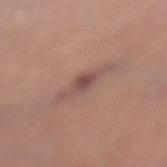Assessment: No biopsy was performed on this lesion — it was imaged during a full skin examination and was not determined to be concerning. Background: The lesion-visualizer software estimated a footprint of about 6 mm², an outline eccentricity of about 0.95 (0 = round, 1 = elongated), and two-axis asymmetry of about 0.2. And it measured an automated nevus-likeness rating near 0 out of 100 and a lesion-detection confidence of about 90/100. A lesion tile, about 15 mm wide, cut from a 3D total-body photograph. Captured under white-light illumination. Measured at roughly 5 mm in maximum diameter. A female patient, aged around 60. From the left lower leg.